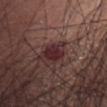biopsy status: no biopsy performed (imaged during a skin exam) | patient: female, aged around 65 | lighting: white-light | body site: the head or neck | lesion diameter: about 3 mm | automated lesion analysis: border irregularity of about 2 on a 0–10 scale, a within-lesion color-variation index near 3/10, and radial color variation of about 1 | imaging modality: total-body-photography crop, ~15 mm field of view.